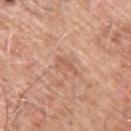<case>
<biopsy_status>not biopsied; imaged during a skin examination</biopsy_status>
<patient>
  <sex>male</sex>
  <age_approx>60</age_approx>
</patient>
<site>right upper arm</site>
<lesion_size>
  <long_diameter_mm_approx>3.5</long_diameter_mm_approx>
</lesion_size>
<lighting>white-light</lighting>
<automated_metrics>
  <area_mm2_approx>3.0</area_mm2_approx>
  <eccentricity>0.9</eccentricity>
  <cielab_L>59</cielab_L>
  <cielab_a>22</cielab_a>
  <cielab_b>32</cielab_b>
  <vs_skin_darker_L>8.0</vs_skin_darker_L>
  <vs_skin_contrast_norm>5.0</vs_skin_contrast_norm>
  <border_irregularity_0_10>5.0</border_irregularity_0_10>
  <color_variation_0_10>1.0</color_variation_0_10>
</automated_metrics>
<image>
  <source>total-body photography crop</source>
  <field_of_view_mm>15</field_of_view_mm>
</image>
</case>